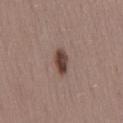Case summary:
– workup: imaged on a skin check; not biopsied
– acquisition: total-body-photography crop, ~15 mm field of view
– size: ≈3 mm
– automated lesion analysis: a lesion area of about 4.5 mm², an outline eccentricity of about 0.8 (0 = round, 1 = elongated), and a shape-asymmetry score of about 0.2 (0 = symmetric); a lesion color around L≈41 a*≈18 b*≈21 in CIELAB and about 15 CIELAB-L* units darker than the surrounding skin
– tile lighting: white-light illumination
– patient: female, roughly 45 years of age
– location: the lower back